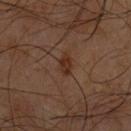Q: Was a biopsy performed?
A: total-body-photography surveillance lesion; no biopsy
Q: Automated lesion metrics?
A: a mean CIELAB color near L≈27 a*≈18 b*≈25, a lesion–skin lightness drop of about 7, and a normalized lesion–skin contrast near 8.5
Q: What is the anatomic site?
A: the chest
Q: Patient demographics?
A: male, aged approximately 60
Q: Lesion size?
A: ~2.5 mm (longest diameter)
Q: What lighting was used for the tile?
A: cross-polarized illumination
Q: How was this image acquired?
A: ~15 mm tile from a whole-body skin photo